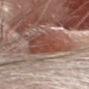Imaged with white-light lighting. A female subject, aged approximately 55. Automated image analysis of the tile measured an area of roughly 19 mm², an outline eccentricity of about 0.65 (0 = round, 1 = elongated), and two-axis asymmetry of about 0.3. It also reported a lesion–skin lightness drop of about 10 and a lesion-to-skin contrast of about 8.5 (normalized; higher = more distinct). And it measured a classifier nevus-likeness of about 100/100 and lesion-presence confidence of about 0/100. The lesion is located on the head or neck. A region of skin cropped from a whole-body photographic capture, roughly 15 mm wide. The lesion's longest dimension is about 6 mm.